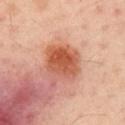Assessment: Imaged during a routine full-body skin examination; the lesion was not biopsied and no histopathology is available. Acquisition and patient details: Cropped from a whole-body photographic skin survey; the tile spans about 15 mm. Located on the left arm. The total-body-photography lesion software estimated a footprint of about 12 mm², an outline eccentricity of about 0.6 (0 = round, 1 = elongated), and two-axis asymmetry of about 0.2. It also reported a mean CIELAB color near L≈53 a*≈29 b*≈35, roughly 12 lightness units darker than nearby skin, and a lesion-to-skin contrast of about 9 (normalized; higher = more distinct). The software also gave a lesion-detection confidence of about 100/100. Measured at roughly 4.5 mm in maximum diameter. A male subject aged approximately 50.